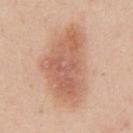Q: Was this lesion biopsied?
A: no biopsy performed (imaged during a skin exam)
Q: Who is the patient?
A: male, approximately 45 years of age
Q: How was this image acquired?
A: ~15 mm tile from a whole-body skin photo
Q: Lesion location?
A: the chest
Q: Illumination type?
A: white-light illumination